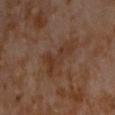Notes:
– follow-up: imaged on a skin check; not biopsied
– subject: male, aged around 60
– lighting: cross-polarized
– imaging modality: ~15 mm tile from a whole-body skin photo
– TBP lesion metrics: a lesion area of about 8 mm² and an outline eccentricity of about 0.85 (0 = round, 1 = elongated); a border-irregularity rating of about 7.5/10, a within-lesion color-variation index near 2.5/10, and a peripheral color-asymmetry measure near 0.5
– size: ≈4.5 mm
– anatomic site: the chest